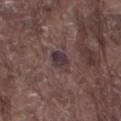notes: no biopsy performed (imaged during a skin exam) | anatomic site: the left thigh | patient: male, in their 80s | image: total-body-photography crop, ~15 mm field of view.